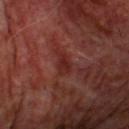The lesion was photographed on a routine skin check and not biopsied; there is no pathology result.
The lesion is located on the chest.
Automated image analysis of the tile measured a lesion area of about 4 mm², an outline eccentricity of about 0.75 (0 = round, 1 = elongated), and a shape-asymmetry score of about 0.35 (0 = symmetric). The analysis additionally found peripheral color asymmetry of about 1. The software also gave a nevus-likeness score of about 0/100.
The patient is a male aged 68 to 72.
Captured under cross-polarized illumination.
Longest diameter approximately 3 mm.
A 15 mm close-up tile from a total-body photography series done for melanoma screening.